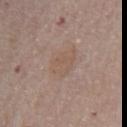• follow-up — imaged on a skin check; not biopsied
• lesion size — about 3.5 mm
• automated lesion analysis — an average lesion color of about L≈54 a*≈15 b*≈26 (CIELAB), a lesion–skin lightness drop of about 5, and a normalized border contrast of about 4.5
• location — the mid back
• illumination — white-light
• subject — male, approximately 75 years of age
• imaging modality — 15 mm crop, total-body photography